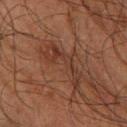{"biopsy_status": "not biopsied; imaged during a skin examination", "lesion_size": {"long_diameter_mm_approx": 6.0}, "lighting": "cross-polarized", "patient": {"sex": "male", "age_approx": 65}, "automated_metrics": {"area_mm2_approx": 7.5, "eccentricity": 0.95, "shape_asymmetry": 0.7, "border_irregularity_0_10": 10.0, "color_variation_0_10": 3.5, "peripheral_color_asymmetry": 1.5}, "image": {"source": "total-body photography crop", "field_of_view_mm": 15}, "site": "leg"}